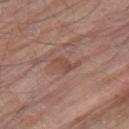Assessment:
Captured during whole-body skin photography for melanoma surveillance; the lesion was not biopsied.